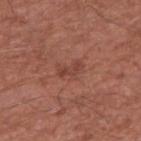Assessment: The lesion was photographed on a routine skin check and not biopsied; there is no pathology result. Acquisition and patient details: Located on the right upper arm. A male subject, in their mid-60s. Captured under white-light illumination. A region of skin cropped from a whole-body photographic capture, roughly 15 mm wide. Measured at roughly 3 mm in maximum diameter. Automated image analysis of the tile measured a nevus-likeness score of about 0/100 and lesion-presence confidence of about 100/100.